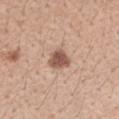Recorded during total-body skin imaging; not selected for excision or biopsy.
A female patient, aged around 45.
A region of skin cropped from a whole-body photographic capture, roughly 15 mm wide.
Longest diameter approximately 3 mm.
The lesion is located on the arm.